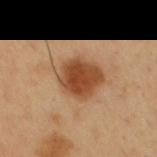follow-up: catalogued during a skin exam; not biopsied | tile lighting: cross-polarized illumination | anatomic site: the abdomen | subject: male, about 50 years old | imaging modality: total-body-photography crop, ~15 mm field of view | diameter: ~2.5 mm (longest diameter).